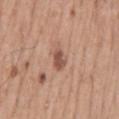Clinical impression:
This lesion was catalogued during total-body skin photography and was not selected for biopsy.
Acquisition and patient details:
A male patient, about 55 years old. About 2.5 mm across. From the mid back. Cropped from a total-body skin-imaging series; the visible field is about 15 mm. Captured under white-light illumination. Automated image analysis of the tile measured a border-irregularity index near 2.5/10, a color-variation rating of about 1.5/10, and a peripheral color-asymmetry measure near 0.5. The analysis additionally found a detector confidence of about 100 out of 100 that the crop contains a lesion.